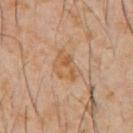Recorded during total-body skin imaging; not selected for excision or biopsy.
The patient is a male approximately 60 years of age.
On the chest.
A lesion tile, about 15 mm wide, cut from a 3D total-body photograph.
The lesion's longest dimension is about 4 mm.